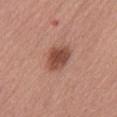| feature | finding |
|---|---|
| follow-up | imaged on a skin check; not biopsied |
| size | ~4 mm (longest diameter) |
| subject | male, in their 70s |
| anatomic site | the front of the torso |
| acquisition | 15 mm crop, total-body photography |
| tile lighting | white-light illumination |